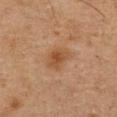The lesion was tiled from a total-body skin photograph and was not biopsied. This is a cross-polarized tile. From the abdomen. Measured at roughly 3 mm in maximum diameter. A male subject aged 73–77. A 15 mm close-up extracted from a 3D total-body photography capture.